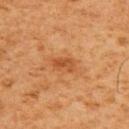{"biopsy_status": "not biopsied; imaged during a skin examination", "automated_metrics": {"cielab_L": 42, "cielab_a": 24, "cielab_b": 35, "vs_skin_darker_L": 8.0, "border_irregularity_0_10": 2.5, "color_variation_0_10": 2.5, "peripheral_color_asymmetry": 1.0, "nevus_likeness_0_100": 35, "lesion_detection_confidence_0_100": 100}, "patient": {"sex": "male", "age_approx": 60}, "lighting": "cross-polarized", "site": "upper back", "image": {"source": "total-body photography crop", "field_of_view_mm": 15}, "lesion_size": {"long_diameter_mm_approx": 2.5}}